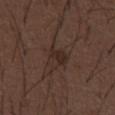Imaged during a routine full-body skin examination; the lesion was not biopsied and no histopathology is available.
A roughly 15 mm field-of-view crop from a total-body skin photograph.
A male subject roughly 50 years of age.
Approximately 3.5 mm at its widest.
Imaged with white-light lighting.
On the front of the torso.